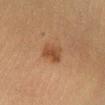No biopsy was performed on this lesion — it was imaged during a full skin examination and was not determined to be concerning. Measured at roughly 3.5 mm in maximum diameter. An algorithmic analysis of the crop reported a footprint of about 6 mm², an outline eccentricity of about 0.75 (0 = round, 1 = elongated), and a symmetry-axis asymmetry near 0.2. The software also gave a mean CIELAB color near L≈41 a*≈19 b*≈31, roughly 9 lightness units darker than nearby skin, and a lesion-to-skin contrast of about 7.5 (normalized; higher = more distinct). The analysis additionally found border irregularity of about 2 on a 0–10 scale, a within-lesion color-variation index near 2.5/10, and a peripheral color-asymmetry measure near 1. Imaged with cross-polarized lighting. A female subject aged approximately 40. A 15 mm crop from a total-body photograph taken for skin-cancer surveillance. From the left lower leg.